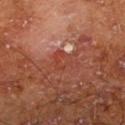Clinical impression: Recorded during total-body skin imaging; not selected for excision or biopsy. Image and clinical context: A male patient, in their mid-60s. On the left lower leg. This is a cross-polarized tile. Approximately 3.5 mm at its widest. Automated image analysis of the tile measured border irregularity of about 9 on a 0–10 scale and internal color variation of about 0 on a 0–10 scale. A close-up tile cropped from a whole-body skin photograph, about 15 mm across.